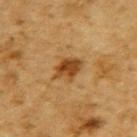Acquisition and patient details: Located on the upper back. The subject is a male aged around 85 or older. A 15 mm close-up tile from a total-body photography series done for melanoma screening.